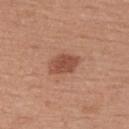The lesion was photographed on a routine skin check and not biopsied; there is no pathology result.
A 15 mm crop from a total-body photograph taken for skin-cancer surveillance.
From the upper back.
The total-body-photography lesion software estimated border irregularity of about 2 on a 0–10 scale and a within-lesion color-variation index near 2/10.
Approximately 3.5 mm at its widest.
A male patient, aged 53 to 57.